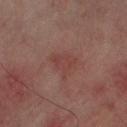Captured during whole-body skin photography for melanoma surveillance; the lesion was not biopsied.
From the right forearm.
A region of skin cropped from a whole-body photographic capture, roughly 15 mm wide.
A male subject about 60 years old.